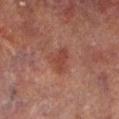follow-up: imaged on a skin check; not biopsied | image source: total-body-photography crop, ~15 mm field of view | automated metrics: a lesion area of about 7 mm², an outline eccentricity of about 0.5 (0 = round, 1 = elongated), and a symmetry-axis asymmetry near 0.45; a lesion color around L≈35 a*≈21 b*≈23 in CIELAB, roughly 6 lightness units darker than nearby skin, and a normalized lesion–skin contrast near 6; a border-irregularity index near 4/10, internal color variation of about 2 on a 0–10 scale, and radial color variation of about 0.5; a nevus-likeness score of about 5/100 and a detector confidence of about 100 out of 100 that the crop contains a lesion | patient: male, aged around 70 | location: the left lower leg | tile lighting: cross-polarized | diameter: ~3 mm (longest diameter).